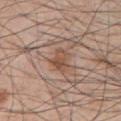Q: Was a biopsy performed?
A: imaged on a skin check; not biopsied
Q: What are the patient's age and sex?
A: male, about 70 years old
Q: Automated lesion metrics?
A: border irregularity of about 3 on a 0–10 scale, a within-lesion color-variation index near 4/10, and a peripheral color-asymmetry measure near 1.5; a classifier nevus-likeness of about 25/100 and a detector confidence of about 100 out of 100 that the crop contains a lesion
Q: Lesion size?
A: ~3 mm (longest diameter)
Q: How was this image acquired?
A: ~15 mm tile from a whole-body skin photo
Q: Lesion location?
A: the front of the torso